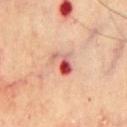Part of a total-body skin-imaging series; this lesion was reviewed on a skin check and was not flagged for biopsy.